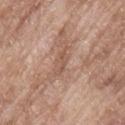| feature | finding |
|---|---|
| follow-up | no biopsy performed (imaged during a skin exam) |
| subject | male, in their mid-60s |
| site | the upper back |
| image source | ~15 mm tile from a whole-body skin photo |